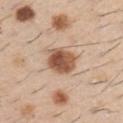{
  "biopsy_status": "not biopsied; imaged during a skin examination",
  "automated_metrics": {
    "cielab_L": 54,
    "cielab_a": 21,
    "cielab_b": 31,
    "vs_skin_darker_L": 16.0,
    "nevus_likeness_0_100": 100,
    "lesion_detection_confidence_0_100": 100
  },
  "patient": {
    "sex": "male",
    "age_approx": 60
  },
  "lighting": "white-light",
  "lesion_size": {
    "long_diameter_mm_approx": 3.5
  },
  "image": {
    "source": "total-body photography crop",
    "field_of_view_mm": 15
  },
  "site": "right upper arm"
}